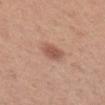{"biopsy_status": "not biopsied; imaged during a skin examination", "site": "arm", "lighting": "white-light", "patient": {"sex": "female", "age_approx": 30}, "lesion_size": {"long_diameter_mm_approx": 2.5}, "image": {"source": "total-body photography crop", "field_of_view_mm": 15}}